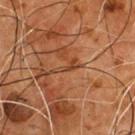| field | value |
|---|---|
| workup | total-body-photography surveillance lesion; no biopsy |
| location | the chest |
| patient | male, in their mid-50s |
| diameter | ≈3 mm |
| image | ~15 mm crop, total-body skin-cancer survey |